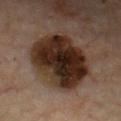{"automated_metrics": {"cielab_L": 27, "cielab_a": 16, "cielab_b": 23, "vs_skin_contrast_norm": 15.5, "color_variation_0_10": 8.5, "peripheral_color_asymmetry": 3.0}, "patient": {"age_approx": 65}, "image": {"source": "total-body photography crop", "field_of_view_mm": 15}, "site": "chest", "lesion_size": {"long_diameter_mm_approx": 7.5}, "lighting": "cross-polarized"}